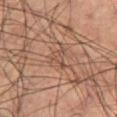Findings:
- notes: total-body-photography surveillance lesion; no biopsy
- location: the right thigh
- diameter: about 3 mm
- illumination: cross-polarized
- subject: male, aged approximately 60
- imaging modality: ~15 mm crop, total-body skin-cancer survey